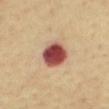Clinical impression:
This lesion was catalogued during total-body skin photography and was not selected for biopsy.
Image and clinical context:
The subject is a male aged approximately 55. Measured at roughly 4 mm in maximum diameter. From the mid back. Cropped from a whole-body photographic skin survey; the tile spans about 15 mm. The lesion-visualizer software estimated a border-irregularity rating of about 1.5/10 and a peripheral color-asymmetry measure near 1.5. The software also gave a nevus-likeness score of about 80/100 and a detector confidence of about 100 out of 100 that the crop contains a lesion. Imaged with cross-polarized lighting.